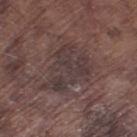Q: What is the lesion's diameter?
A: ~6.5 mm (longest diameter)
Q: What kind of image is this?
A: 15 mm crop, total-body photography
Q: What did automated image analysis measure?
A: an average lesion color of about L≈36 a*≈14 b*≈15 (CIELAB); a nevus-likeness score of about 0/100 and lesion-presence confidence of about 90/100
Q: What is the anatomic site?
A: the right thigh
Q: Illumination type?
A: white-light illumination
Q: Patient demographics?
A: male, aged approximately 75